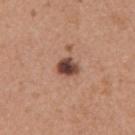– follow-up: imaged on a skin check; not biopsied
– subject: female, aged approximately 35
– automated lesion analysis: a lesion color around L≈45 a*≈22 b*≈26 in CIELAB, about 17 CIELAB-L* units darker than the surrounding skin, and a lesion-to-skin contrast of about 12.5 (normalized; higher = more distinct); a border-irregularity rating of about 2/10 and a color-variation rating of about 5/10; an automated nevus-likeness rating near 70 out of 100 and lesion-presence confidence of about 100/100
– image: ~15 mm crop, total-body skin-cancer survey
– body site: the left upper arm